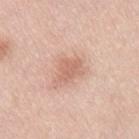follow-up: imaged on a skin check; not biopsied
patient: female, in their 60s
anatomic site: the lower back
size: about 2.5 mm
acquisition: ~15 mm crop, total-body skin-cancer survey
lighting: white-light illumination